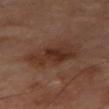biopsy status: imaged on a skin check; not biopsied | tile lighting: cross-polarized | body site: the right thigh | lesion size: ~7 mm (longest diameter) | image: total-body-photography crop, ~15 mm field of view | patient: male, aged 68 to 72.